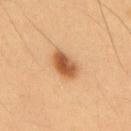notes — no biopsy performed (imaged during a skin exam) | body site — the upper back | tile lighting — cross-polarized | subject — male, aged 53–57 | image — ~15 mm tile from a whole-body skin photo | automated lesion analysis — an area of roughly 7.5 mm², an outline eccentricity of about 0.8 (0 = round, 1 = elongated), and two-axis asymmetry of about 0.15; internal color variation of about 5 on a 0–10 scale and peripheral color asymmetry of about 1.5; an automated nevus-likeness rating near 100 out of 100 and lesion-presence confidence of about 100/100.